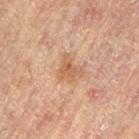The subject is a female aged around 80. Measured at roughly 3 mm in maximum diameter. On the right leg. The tile uses cross-polarized illumination. A region of skin cropped from a whole-body photographic capture, roughly 15 mm wide.